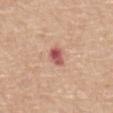| field | value |
|---|---|
| notes | imaged on a skin check; not biopsied |
| body site | the mid back |
| acquisition | ~15 mm tile from a whole-body skin photo |
| patient | male, roughly 70 years of age |
| illumination | white-light illumination |
| diameter | ~3 mm (longest diameter) |
| automated lesion analysis | an area of roughly 4 mm² and a shape eccentricity near 0.75; roughly 14 lightness units darker than nearby skin and a lesion-to-skin contrast of about 9.5 (normalized; higher = more distinct) |